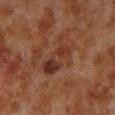Q: Was this lesion biopsied?
A: imaged on a skin check; not biopsied
Q: What lighting was used for the tile?
A: cross-polarized illumination
Q: What is the imaging modality?
A: total-body-photography crop, ~15 mm field of view
Q: Automated lesion metrics?
A: a mean CIELAB color near L≈34 a*≈22 b*≈28, about 8 CIELAB-L* units darker than the surrounding skin, and a normalized border contrast of about 7; a within-lesion color-variation index near 6/10 and a peripheral color-asymmetry measure near 2
Q: How large is the lesion?
A: ≈5 mm
Q: Patient demographics?
A: male, about 70 years old
Q: What is the anatomic site?
A: the left lower leg